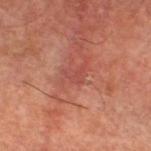{"biopsy_status": "not biopsied; imaged during a skin examination", "lesion_size": {"long_diameter_mm_approx": 2.5}, "patient": {"sex": "male", "age_approx": 60}, "image": {"source": "total-body photography crop", "field_of_view_mm": 15}, "site": "leg", "lighting": "cross-polarized", "automated_metrics": {"area_mm2_approx": 2.5, "eccentricity": 0.7, "shape_asymmetry": 0.75, "cielab_L": 45, "cielab_a": 29, "cielab_b": 27, "vs_skin_darker_L": 6.0, "vs_skin_contrast_norm": 4.5, "border_irregularity_0_10": 7.5, "color_variation_0_10": 0.0, "peripheral_color_asymmetry": 0.0, "nevus_likeness_0_100": 0, "lesion_detection_confidence_0_100": 100}}